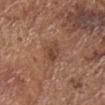Clinical impression:
The lesion was tiled from a total-body skin photograph and was not biopsied.
Acquisition and patient details:
This image is a 15 mm lesion crop taken from a total-body photograph. The patient is a male aged around 75. From the head or neck. Longest diameter approximately 4 mm.